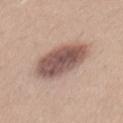{"image": {"source": "total-body photography crop", "field_of_view_mm": 15}, "automated_metrics": {"area_mm2_approx": 21.0, "eccentricity": 0.8, "vs_skin_darker_L": 16.0, "vs_skin_contrast_norm": 10.5, "nevus_likeness_0_100": 65, "lesion_detection_confidence_0_100": 100}, "patient": {"sex": "male", "age_approx": 25}, "lesion_size": {"long_diameter_mm_approx": 7.0}, "site": "mid back", "lighting": "white-light"}